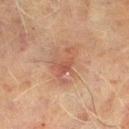notes=imaged on a skin check; not biopsied
lighting=cross-polarized illumination
site=the right lower leg
patient=male, in their 70s
acquisition=~15 mm tile from a whole-body skin photo
size=about 4.5 mm